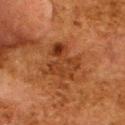This lesion was catalogued during total-body skin photography and was not selected for biopsy. The tile uses cross-polarized illumination. Automated tile analysis of the lesion measured a border-irregularity index near 6/10, a color-variation rating of about 6/10, and a peripheral color-asymmetry measure near 2. A female subject, roughly 50 years of age. The lesion's longest dimension is about 4.5 mm. A close-up tile cropped from a whole-body skin photograph, about 15 mm across. The lesion is located on the upper back.